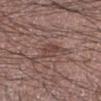Captured during whole-body skin photography for melanoma surveillance; the lesion was not biopsied. The total-body-photography lesion software estimated internal color variation of about 2 on a 0–10 scale and radial color variation of about 0.5. The analysis additionally found an automated nevus-likeness rating near 0 out of 100 and a lesion-detection confidence of about 60/100. A male subject, in their mid- to late 60s. Located on the right forearm. A close-up tile cropped from a whole-body skin photograph, about 15 mm across. The tile uses white-light illumination.